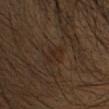Part of a total-body skin-imaging series; this lesion was reviewed on a skin check and was not flagged for biopsy. About 3 mm across. The tile uses cross-polarized illumination. A male subject aged 53–57. A 15 mm close-up extracted from a 3D total-body photography capture. The total-body-photography lesion software estimated a shape eccentricity near 0.9 and a shape-asymmetry score of about 0.4 (0 = symmetric). The lesion is located on the head or neck.